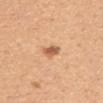Captured during whole-body skin photography for melanoma surveillance; the lesion was not biopsied. A female subject, aged around 45. Captured under white-light illumination. Approximately 2.5 mm at its widest. Cropped from a whole-body photographic skin survey; the tile spans about 15 mm. The lesion is on the upper back.